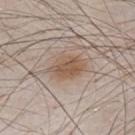{"biopsy_status": "not biopsied; imaged during a skin examination", "lighting": "white-light", "automated_metrics": {"area_mm2_approx": 10.0, "eccentricity": 0.8, "shape_asymmetry": 0.2, "cielab_L": 55, "cielab_a": 15, "cielab_b": 28, "vs_skin_darker_L": 9.0, "vs_skin_contrast_norm": 7.5, "nevus_likeness_0_100": 100}, "site": "abdomen", "image": {"source": "total-body photography crop", "field_of_view_mm": 15}, "patient": {"sex": "male", "age_approx": 80}, "lesion_size": {"long_diameter_mm_approx": 5.0}}